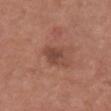Q: Is there a histopathology result?
A: catalogued during a skin exam; not biopsied
Q: What is the imaging modality?
A: 15 mm crop, total-body photography
Q: Who is the patient?
A: female, aged 48–52
Q: How large is the lesion?
A: ~3.5 mm (longest diameter)
Q: What lighting was used for the tile?
A: white-light illumination
Q: What is the anatomic site?
A: the chest